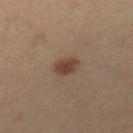Q: Is there a histopathology result?
A: imaged on a skin check; not biopsied
Q: What are the patient's age and sex?
A: female, approximately 40 years of age
Q: Automated lesion metrics?
A: a lesion area of about 4.5 mm², a shape eccentricity near 0.75, and a symmetry-axis asymmetry near 0.25; a border-irregularity index near 2/10 and internal color variation of about 2.5 on a 0–10 scale; a classifier nevus-likeness of about 95/100 and a lesion-detection confidence of about 100/100
Q: What is the anatomic site?
A: the left leg
Q: How was the tile lit?
A: cross-polarized
Q: What kind of image is this?
A: ~15 mm crop, total-body skin-cancer survey
Q: Lesion size?
A: ~3 mm (longest diameter)